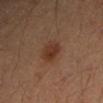The lesion was tiled from a total-body skin photograph and was not biopsied. The lesion-visualizer software estimated a nevus-likeness score of about 95/100 and lesion-presence confidence of about 100/100. Cropped from a whole-body photographic skin survey; the tile spans about 15 mm. A female subject, about 35 years old. The lesion is located on the left forearm.